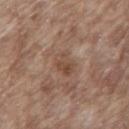Recorded during total-body skin imaging; not selected for excision or biopsy. On the mid back. The patient is a male approximately 65 years of age. This image is a 15 mm lesion crop taken from a total-body photograph.